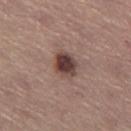The lesion was photographed on a routine skin check and not biopsied; there is no pathology result.
The total-body-photography lesion software estimated an average lesion color of about L≈42 a*≈18 b*≈21 (CIELAB), roughly 14 lightness units darker than nearby skin, and a normalized border contrast of about 11.
Measured at roughly 3.5 mm in maximum diameter.
A female patient, aged around 65.
The tile uses white-light illumination.
Cropped from a whole-body photographic skin survey; the tile spans about 15 mm.
The lesion is on the right thigh.